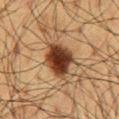follow-up: catalogued during a skin exam; not biopsied.